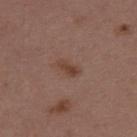Impression: Part of a total-body skin-imaging series; this lesion was reviewed on a skin check and was not flagged for biopsy. Image and clinical context: A 15 mm close-up tile from a total-body photography series done for melanoma screening. A female subject aged around 45. The total-body-photography lesion software estimated a mean CIELAB color near L≈41 a*≈19 b*≈26, roughly 8 lightness units darker than nearby skin, and a normalized lesion–skin contrast near 7.5. Captured under white-light illumination. The lesion is located on the upper back.